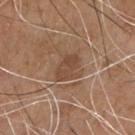<case>
<biopsy_status>not biopsied; imaged during a skin examination</biopsy_status>
<lighting>white-light</lighting>
<image>
  <source>total-body photography crop</source>
  <field_of_view_mm>15</field_of_view_mm>
</image>
<lesion_size>
  <long_diameter_mm_approx>3.5</long_diameter_mm_approx>
</lesion_size>
<site>chest</site>
<patient>
  <sex>male</sex>
  <age_approx>70</age_approx>
</patient>
<automated_metrics>
  <area_mm2_approx>6.0</area_mm2_approx>
  <eccentricity>0.8</eccentricity>
  <shape_asymmetry>0.2</shape_asymmetry>
  <vs_skin_darker_L>8.0</vs_skin_darker_L>
  <vs_skin_contrast_norm>6.5</vs_skin_contrast_norm>
  <border_irregularity_0_10>2.5</border_irregularity_0_10>
  <color_variation_0_10>3.0</color_variation_0_10>
</automated_metrics>
</case>